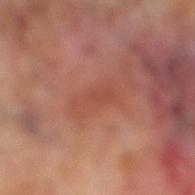notes: catalogued during a skin exam; not biopsied.